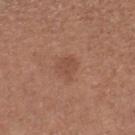Case summary:
– tile lighting: white-light illumination
– anatomic site: the right lower leg
– automated metrics: a mean CIELAB color near L≈48 a*≈22 b*≈29, about 7 CIELAB-L* units darker than the surrounding skin, and a normalized lesion–skin contrast near 5; lesion-presence confidence of about 100/100
– image: ~15 mm crop, total-body skin-cancer survey
– subject: female, in their mid-50s